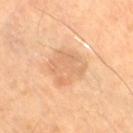{
  "biopsy_status": "not biopsied; imaged during a skin examination",
  "patient": {
    "age_approx": 65
  },
  "image": {
    "source": "total-body photography crop",
    "field_of_view_mm": 15
  },
  "automated_metrics": {
    "shape_asymmetry": 0.2,
    "cielab_L": 68,
    "cielab_a": 21,
    "cielab_b": 37,
    "vs_skin_darker_L": 8.0,
    "nevus_likeness_0_100": 10,
    "lesion_detection_confidence_0_100": 100
  },
  "site": "right thigh",
  "lighting": "cross-polarized",
  "lesion_size": {
    "long_diameter_mm_approx": 4.5
  }
}